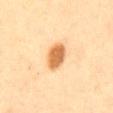Case summary:
* lesion diameter: ~4 mm (longest diameter)
* location: the chest
* TBP lesion metrics: an area of roughly 7.5 mm², an outline eccentricity of about 0.75 (0 = round, 1 = elongated), and two-axis asymmetry of about 0.1; a mean CIELAB color near L≈57 a*≈20 b*≈39, about 14 CIELAB-L* units darker than the surrounding skin, and a lesion-to-skin contrast of about 9.5 (normalized; higher = more distinct); a border-irregularity index near 1/10, a within-lesion color-variation index near 2.5/10, and a peripheral color-asymmetry measure near 1; an automated nevus-likeness rating near 100 out of 100 and a detector confidence of about 100 out of 100 that the crop contains a lesion
* image: total-body-photography crop, ~15 mm field of view
* subject: female, about 55 years old